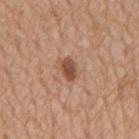follow-up: no biopsy performed (imaged during a skin exam); subject: male, approximately 65 years of age; acquisition: 15 mm crop, total-body photography; location: the mid back.